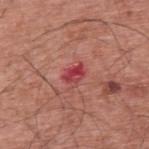Part of a total-body skin-imaging series; this lesion was reviewed on a skin check and was not flagged for biopsy.
Approximately 2.5 mm at its widest.
A 15 mm close-up extracted from a 3D total-body photography capture.
A male subject aged 58–62.
From the upper back.
This is a white-light tile.